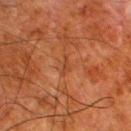Assessment:
Imaged during a routine full-body skin examination; the lesion was not biopsied and no histopathology is available.
Context:
Approximately 2.5 mm at its widest. A 15 mm close-up tile from a total-body photography series done for melanoma screening. The lesion is on the left lower leg. A male subject, about 80 years old. Imaged with cross-polarized lighting.